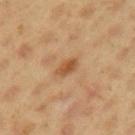This lesion was catalogued during total-body skin photography and was not selected for biopsy. The tile uses cross-polarized illumination. A 15 mm close-up extracted from a 3D total-body photography capture. On the left upper arm. The subject is a male approximately 45 years of age. About 3 mm across.